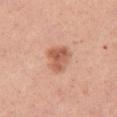This lesion was catalogued during total-body skin photography and was not selected for biopsy.
A female patient about 30 years old.
Located on the right thigh.
About 3.5 mm across.
Captured under white-light illumination.
A 15 mm close-up extracted from a 3D total-body photography capture.
Automated image analysis of the tile measured a border-irregularity rating of about 3.5/10, a color-variation rating of about 4/10, and a peripheral color-asymmetry measure near 1.5.